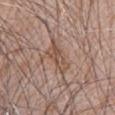This lesion was catalogued during total-body skin photography and was not selected for biopsy. This image is a 15 mm lesion crop taken from a total-body photograph. On the abdomen. The subject is a male aged approximately 60. The lesion's longest dimension is about 4.5 mm.